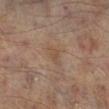Impression:
The lesion was photographed on a routine skin check and not biopsied; there is no pathology result.
Context:
The lesion is located on the right lower leg. The lesion's longest dimension is about 3 mm. A male patient aged approximately 65. Cropped from a total-body skin-imaging series; the visible field is about 15 mm. This is a cross-polarized tile.